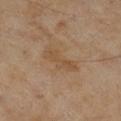Impression:
Recorded during total-body skin imaging; not selected for excision or biopsy.